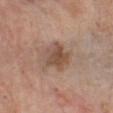{"biopsy_status": "not biopsied; imaged during a skin examination", "lighting": "white-light", "lesion_size": {"long_diameter_mm_approx": 4.0}, "automated_metrics": {"area_mm2_approx": 10.0, "eccentricity": 0.6, "shape_asymmetry": 0.25, "cielab_L": 51, "cielab_a": 18, "cielab_b": 27, "vs_skin_darker_L": 10.0, "vs_skin_contrast_norm": 7.5, "border_irregularity_0_10": 2.5, "color_variation_0_10": 5.5, "nevus_likeness_0_100": 15, "lesion_detection_confidence_0_100": 100}, "patient": {"sex": "male", "age_approx": 60}, "site": "chest", "image": {"source": "total-body photography crop", "field_of_view_mm": 15}}